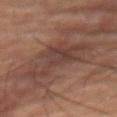No biopsy was performed on this lesion — it was imaged during a full skin examination and was not determined to be concerning.
The tile uses cross-polarized illumination.
Cropped from a total-body skin-imaging series; the visible field is about 15 mm.
A male subject, aged 53–57.
The lesion is on the abdomen.